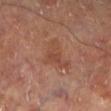Case summary:
• workup: imaged on a skin check; not biopsied
• tile lighting: cross-polarized
• site: the left lower leg
• image source: 15 mm crop, total-body photography
• patient: male, aged around 70
• lesion size: ~4 mm (longest diameter)